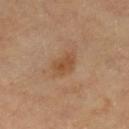follow-up — total-body-photography surveillance lesion; no biopsy | lesion size — ~4 mm (longest diameter) | location — the leg | imaging modality — total-body-photography crop, ~15 mm field of view | subject — female, in their 60s | TBP lesion metrics — a lesion area of about 6 mm² and two-axis asymmetry of about 0.25; a lesion color around L≈51 a*≈20 b*≈35 in CIELAB, a lesion–skin lightness drop of about 8, and a lesion-to-skin contrast of about 7 (normalized; higher = more distinct); lesion-presence confidence of about 100/100.